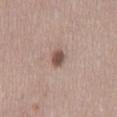Impression: Imaged during a routine full-body skin examination; the lesion was not biopsied and no histopathology is available. Background: A region of skin cropped from a whole-body photographic capture, roughly 15 mm wide. Located on the mid back. A female subject, aged around 40.